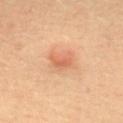No biopsy was performed on this lesion — it was imaged during a full skin examination and was not determined to be concerning.
Imaged with cross-polarized lighting.
A close-up tile cropped from a whole-body skin photograph, about 15 mm across.
Measured at roughly 3 mm in maximum diameter.
The patient is a female aged approximately 35.
On the upper back.